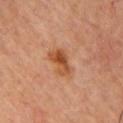notes: total-body-photography surveillance lesion; no biopsy
size: ~4 mm (longest diameter)
anatomic site: the chest
acquisition: ~15 mm crop, total-body skin-cancer survey
subject: male, aged approximately 45
tile lighting: cross-polarized illumination